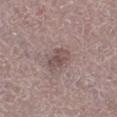{"biopsy_status": "not biopsied; imaged during a skin examination", "automated_metrics": {"area_mm2_approx": 6.5, "eccentricity": 0.75, "shape_asymmetry": 0.2, "vs_skin_darker_L": 9.0, "vs_skin_contrast_norm": 6.5, "border_irregularity_0_10": 2.0, "color_variation_0_10": 3.0, "peripheral_color_asymmetry": 1.0}, "image": {"source": "total-body photography crop", "field_of_view_mm": 15}, "lesion_size": {"long_diameter_mm_approx": 3.5}, "patient": {"sex": "male", "age_approx": 65}, "lighting": "white-light", "site": "left lower leg"}